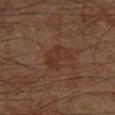Q: Was a biopsy performed?
A: imaged on a skin check; not biopsied
Q: What lighting was used for the tile?
A: cross-polarized illumination
Q: What kind of image is this?
A: total-body-photography crop, ~15 mm field of view
Q: What is the anatomic site?
A: the right lower leg
Q: What are the patient's age and sex?
A: male, in their 60s